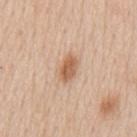{"biopsy_status": "not biopsied; imaged during a skin examination"}